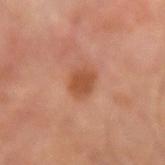| key | value |
|---|---|
| biopsy status | total-body-photography surveillance lesion; no biopsy |
| anatomic site | the left forearm |
| automated metrics | an area of roughly 6 mm² and a shape-asymmetry score of about 0.15 (0 = symmetric); a border-irregularity rating of about 1.5/10, a within-lesion color-variation index near 2/10, and radial color variation of about 1 |
| lesion size | ≈3 mm |
| image | 15 mm crop, total-body photography |
| patient | male, aged 63–67 |